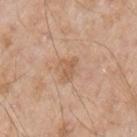Q: Was a biopsy performed?
A: total-body-photography surveillance lesion; no biopsy
Q: What is the anatomic site?
A: the left upper arm
Q: What are the patient's age and sex?
A: male, aged approximately 65
Q: What is the imaging modality?
A: ~15 mm crop, total-body skin-cancer survey